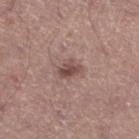Part of a total-body skin-imaging series; this lesion was reviewed on a skin check and was not flagged for biopsy. Measured at roughly 3 mm in maximum diameter. A lesion tile, about 15 mm wide, cut from a 3D total-body photograph. The subject is a male aged 38 to 42. Imaged with white-light lighting. The lesion is on the left lower leg. An algorithmic analysis of the crop reported roughly 11 lightness units darker than nearby skin and a lesion-to-skin contrast of about 8 (normalized; higher = more distinct). It also reported an automated nevus-likeness rating near 70 out of 100 and lesion-presence confidence of about 100/100.